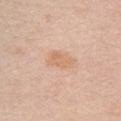Q: Is there a histopathology result?
A: total-body-photography surveillance lesion; no biopsy
Q: How was this image acquired?
A: total-body-photography crop, ~15 mm field of view
Q: Lesion size?
A: about 3.5 mm
Q: Lesion location?
A: the front of the torso
Q: Automated lesion metrics?
A: an average lesion color of about L≈67 a*≈20 b*≈34 (CIELAB) and about 7 CIELAB-L* units darker than the surrounding skin; a border-irregularity index near 3/10, a color-variation rating of about 2.5/10, and peripheral color asymmetry of about 1; a nevus-likeness score of about 0/100 and a lesion-detection confidence of about 100/100
Q: What are the patient's age and sex?
A: male, in their 70s